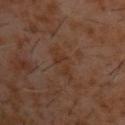The lesion was tiled from a total-body skin photograph and was not biopsied. A lesion tile, about 15 mm wide, cut from a 3D total-body photograph. From the upper back. Captured under cross-polarized illumination. The subject is a male aged 58 to 62. The lesion-visualizer software estimated a lesion color around L≈31 a*≈17 b*≈25 in CIELAB, a lesion–skin lightness drop of about 4, and a normalized border contrast of about 5. The analysis additionally found a border-irregularity index near 5/10, a within-lesion color-variation index near 2/10, and peripheral color asymmetry of about 0.5. The software also gave a classifier nevus-likeness of about 0/100 and a detector confidence of about 100 out of 100 that the crop contains a lesion.